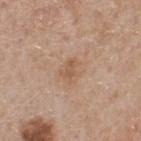Findings:
* workup — total-body-photography surveillance lesion; no biopsy
* tile lighting — white-light
* lesion size — ~2.5 mm (longest diameter)
* image — 15 mm crop, total-body photography
* patient — male, in their mid- to late 50s